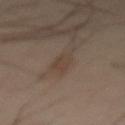Captured during whole-body skin photography for melanoma surveillance; the lesion was not biopsied.
Automated tile analysis of the lesion measured a footprint of about 4 mm², an outline eccentricity of about 0.9 (0 = round, 1 = elongated), and a shape-asymmetry score of about 0.3 (0 = symmetric). The software also gave a peripheral color-asymmetry measure near 1.5.
The lesion is on the abdomen.
The lesion's longest dimension is about 3.5 mm.
This image is a 15 mm lesion crop taken from a total-body photograph.
The patient is a male aged approximately 50.